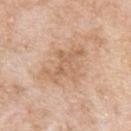Background:
A 15 mm close-up extracted from a 3D total-body photography capture. The lesion is located on the left upper arm. The recorded lesion diameter is about 5.5 mm. The subject is a female in their mid- to late 70s.